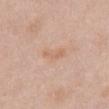follow-up = no biopsy performed (imaged during a skin exam)
size = ~3 mm (longest diameter)
illumination = white-light
image source = ~15 mm crop, total-body skin-cancer survey
patient = female, aged 48–52
location = the chest